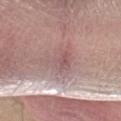Assessment: Part of a total-body skin-imaging series; this lesion was reviewed on a skin check and was not flagged for biopsy. Context: Cropped from a whole-body photographic skin survey; the tile spans about 15 mm. Automated image analysis of the tile measured an average lesion color of about L≈56 a*≈20 b*≈19 (CIELAB), a lesion–skin lightness drop of about 8, and a normalized border contrast of about 5.5. A male subject, in their 80s. Located on the right arm. Approximately 4 mm at its widest. The tile uses white-light illumination.